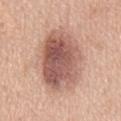Assessment: Imaged during a routine full-body skin examination; the lesion was not biopsied and no histopathology is available. Context: The patient is a male approximately 50 years of age. The lesion is on the mid back. Measured at roughly 8.5 mm in maximum diameter. The tile uses white-light illumination. A lesion tile, about 15 mm wide, cut from a 3D total-body photograph.